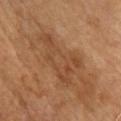Recorded during total-body skin imaging; not selected for excision or biopsy. A region of skin cropped from a whole-body photographic capture, roughly 15 mm wide. Automated image analysis of the tile measured a footprint of about 16 mm², an outline eccentricity of about 0.85 (0 = round, 1 = elongated), and two-axis asymmetry of about 0.55. It also reported a lesion–skin lightness drop of about 7 and a normalized border contrast of about 5.5. And it measured a border-irregularity rating of about 7.5/10 and a peripheral color-asymmetry measure near 1. The subject is a male aged around 65. On the chest. The lesion's longest dimension is about 7.5 mm.